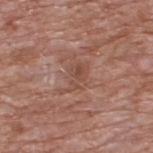{"biopsy_status": "not biopsied; imaged during a skin examination", "patient": {"sex": "male", "age_approx": 60}, "lighting": "white-light", "automated_metrics": {"area_mm2_approx": 5.5, "shape_asymmetry": 0.55, "border_irregularity_0_10": 6.5, "color_variation_0_10": 3.5, "peripheral_color_asymmetry": 1.5, "lesion_detection_confidence_0_100": 100}, "site": "upper back", "image": {"source": "total-body photography crop", "field_of_view_mm": 15}}